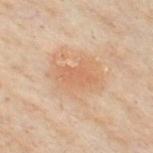notes — catalogued during a skin exam; not biopsied | automated lesion analysis — a footprint of about 6 mm², a shape eccentricity near 0.85, and a shape-asymmetry score of about 0.25 (0 = symmetric); a nevus-likeness score of about 50/100 and a detector confidence of about 100 out of 100 that the crop contains a lesion | diameter — ~4 mm (longest diameter) | subject — male, aged around 50 | anatomic site — the chest | imaging modality — total-body-photography crop, ~15 mm field of view | lighting — cross-polarized illumination.